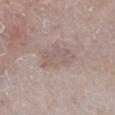follow-up — total-body-photography surveillance lesion; no biopsy
subject — male, approximately 65 years of age
image — total-body-photography crop, ~15 mm field of view
anatomic site — the leg
lighting — white-light illumination
image-analysis metrics — a symmetry-axis asymmetry near 0.3; an average lesion color of about L≈57 a*≈15 b*≈20 (CIELAB) and a lesion-to-skin contrast of about 4.5 (normalized; higher = more distinct); a border-irregularity rating of about 3.5/10, a within-lesion color-variation index near 2/10, and a peripheral color-asymmetry measure near 0.5
diameter — about 5 mm